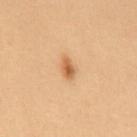The lesion was tiled from a total-body skin photograph and was not biopsied. Cropped from a whole-body photographic skin survey; the tile spans about 15 mm. Imaged with cross-polarized lighting. About 2.5 mm across. The lesion is on the mid back. Automated image analysis of the tile measured an area of roughly 3.5 mm², an eccentricity of roughly 0.8, and a shape-asymmetry score of about 0.25 (0 = symmetric). And it measured a mean CIELAB color near L≈49 a*≈18 b*≈33 and a lesion–skin lightness drop of about 10. And it measured a border-irregularity index near 2/10 and radial color variation of about 1. The software also gave an automated nevus-likeness rating near 95 out of 100. The subject is a female in their 40s.